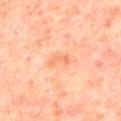Image and clinical context: A region of skin cropped from a whole-body photographic capture, roughly 15 mm wide. The lesion is on the mid back. Measured at roughly 3 mm in maximum diameter. The subject is a male roughly 70 years of age.